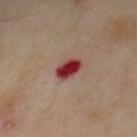workup = total-body-photography surveillance lesion; no biopsy | site = the chest | automated lesion analysis = an area of roughly 5.5 mm² and two-axis asymmetry of about 0.2 | patient = male, aged 68–72 | acquisition = 15 mm crop, total-body photography.